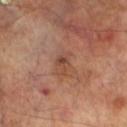biopsy_status: not biopsied; imaged during a skin examination
patient:
  sex: male
  age_approx: 70
lighting: cross-polarized
site: left lower leg
image:
  source: total-body photography crop
  field_of_view_mm: 15
automated_metrics:
  area_mm2_approx: 4.0
  eccentricity: 0.75
  shape_asymmetry: 0.4
  border_irregularity_0_10: 3.5
  color_variation_0_10: 4.0
  peripheral_color_asymmetry: 1.5